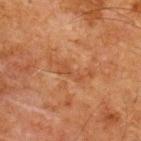This lesion was catalogued during total-body skin photography and was not selected for biopsy. The lesion is on the back. This is a cross-polarized tile. The lesion's longest dimension is about 5.5 mm. A male patient about 65 years old. Cropped from a whole-body photographic skin survey; the tile spans about 15 mm.